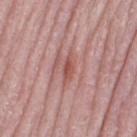Part of a total-body skin-imaging series; this lesion was reviewed on a skin check and was not flagged for biopsy. Measured at roughly 3.5 mm in maximum diameter. The subject is a female aged 63–67. Imaged with white-light lighting. This image is a 15 mm lesion crop taken from a total-body photograph. Automated image analysis of the tile measured an average lesion color of about L≈53 a*≈26 b*≈25 (CIELAB), a lesion–skin lightness drop of about 9, and a lesion-to-skin contrast of about 7 (normalized; higher = more distinct). The analysis additionally found a border-irregularity index near 4/10, a within-lesion color-variation index near 1.5/10, and peripheral color asymmetry of about 0.5. The lesion is located on the leg.